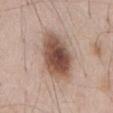Clinical impression:
Captured during whole-body skin photography for melanoma surveillance; the lesion was not biopsied.
Clinical summary:
The lesion is on the abdomen. A region of skin cropped from a whole-body photographic capture, roughly 15 mm wide. The subject is a male aged 53 to 57.